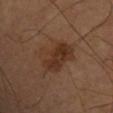<case>
<site>chest</site>
<automated_metrics>
  <shape_asymmetry>0.25</shape_asymmetry>
  <vs_skin_contrast_norm>8.0</vs_skin_contrast_norm>
</automated_metrics>
<patient>
  <sex>male</sex>
  <age_approx>60</age_approx>
</patient>
<lesion_size>
  <long_diameter_mm_approx>6.0</long_diameter_mm_approx>
</lesion_size>
<lighting>cross-polarized</lighting>
<image>
  <source>total-body photography crop</source>
  <field_of_view_mm>15</field_of_view_mm>
</image>
</case>